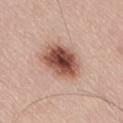Part of a total-body skin-imaging series; this lesion was reviewed on a skin check and was not flagged for biopsy. A male subject, aged 53 to 57. Imaged with white-light lighting. On the leg. Automated tile analysis of the lesion measured a lesion area of about 15 mm², an outline eccentricity of about 0.6 (0 = round, 1 = elongated), and a symmetry-axis asymmetry near 0.15. A 15 mm crop from a total-body photograph taken for skin-cancer surveillance.